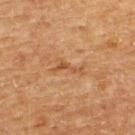This lesion was catalogued during total-body skin photography and was not selected for biopsy. Measured at roughly 4 mm in maximum diameter. From the back. A 15 mm crop from a total-body photograph taken for skin-cancer surveillance. Imaged with cross-polarized lighting. The patient is a male in their mid-60s.